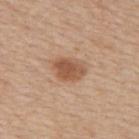Imaged during a routine full-body skin examination; the lesion was not biopsied and no histopathology is available. The lesion is on the back. A region of skin cropped from a whole-body photographic capture, roughly 15 mm wide. A female subject aged 48–52.